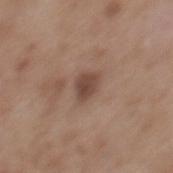| field | value |
|---|---|
| follow-up | no biopsy performed (imaged during a skin exam) |
| image source | ~15 mm tile from a whole-body skin photo |
| tile lighting | white-light illumination |
| lesion size | ≈2.5 mm |
| body site | the back |
| patient | male, aged 53–57 |